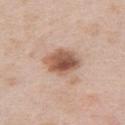Case summary:
* workup · catalogued during a skin exam; not biopsied